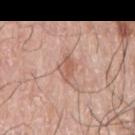follow-up: no biopsy performed (imaged during a skin exam); anatomic site: the left arm; image source: 15 mm crop, total-body photography; lesion size: ≈3 mm; patient: male, aged around 70.